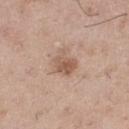follow-up: total-body-photography surveillance lesion; no biopsy
TBP lesion metrics: an outline eccentricity of about 0.6 (0 = round, 1 = elongated) and two-axis asymmetry of about 0.3; a mean CIELAB color near L≈57 a*≈18 b*≈28 and roughly 10 lightness units darker than nearby skin; a border-irregularity index near 3/10, internal color variation of about 3.5 on a 0–10 scale, and radial color variation of about 1.5; a classifier nevus-likeness of about 15/100 and a lesion-detection confidence of about 100/100
image source: total-body-photography crop, ~15 mm field of view
lesion size: ~3 mm (longest diameter)
body site: the right thigh
illumination: white-light
patient: male, aged approximately 50